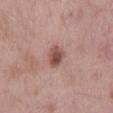Impression: Part of a total-body skin-imaging series; this lesion was reviewed on a skin check and was not flagged for biopsy. Acquisition and patient details: About 3 mm across. A male patient aged around 55. A region of skin cropped from a whole-body photographic capture, roughly 15 mm wide. The lesion is located on the mid back. Captured under white-light illumination.